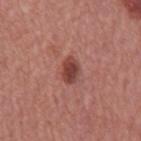Q: What is the imaging modality?
A: ~15 mm crop, total-body skin-cancer survey
Q: Where on the body is the lesion?
A: the mid back
Q: Patient demographics?
A: male, roughly 65 years of age